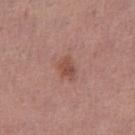Part of a total-body skin-imaging series; this lesion was reviewed on a skin check and was not flagged for biopsy.
The recorded lesion diameter is about 2.5 mm.
A 15 mm close-up extracted from a 3D total-body photography capture.
A female patient, aged 48 to 52.
The lesion is on the leg.
Captured under white-light illumination.